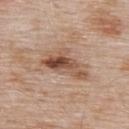biopsy status: catalogued during a skin exam; not biopsied | lesion size: ≈6 mm | illumination: white-light | image-analysis metrics: a lesion area of about 13 mm², a shape eccentricity near 0.85, and a symmetry-axis asymmetry near 0.3; border irregularity of about 4 on a 0–10 scale and a within-lesion color-variation index near 9.5/10 | subject: male, aged 53 to 57 | anatomic site: the upper back | image source: ~15 mm crop, total-body skin-cancer survey.